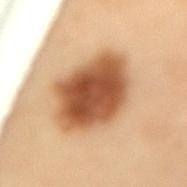| key | value |
|---|---|
| notes | catalogued during a skin exam; not biopsied |
| lesion diameter | about 8 mm |
| acquisition | ~15 mm tile from a whole-body skin photo |
| anatomic site | the back |
| patient | female, approximately 50 years of age |
| lighting | cross-polarized illumination |